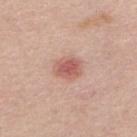Clinical impression:
Part of a total-body skin-imaging series; this lesion was reviewed on a skin check and was not flagged for biopsy.
Acquisition and patient details:
Approximately 3.5 mm at its widest. A female subject, about 55 years old. On the upper back. A close-up tile cropped from a whole-body skin photograph, about 15 mm across.